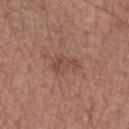biopsy status: imaged on a skin check; not biopsied
automated lesion analysis: an average lesion color of about L≈47 a*≈22 b*≈27 (CIELAB), a lesion–skin lightness drop of about 7, and a lesion-to-skin contrast of about 5.5 (normalized; higher = more distinct); a border-irregularity rating of about 4/10 and internal color variation of about 2 on a 0–10 scale
lighting: white-light
size: ≈3.5 mm
subject: male, in their mid-50s
imaging modality: 15 mm crop, total-body photography
location: the left forearm